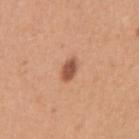Recorded during total-body skin imaging; not selected for excision or biopsy.
Located on the right upper arm.
A female subject, aged around 45.
Cropped from a whole-body photographic skin survey; the tile spans about 15 mm.